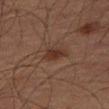{"biopsy_status": "not biopsied; imaged during a skin examination", "image": {"source": "total-body photography crop", "field_of_view_mm": 15}, "lesion_size": {"long_diameter_mm_approx": 3.0}, "lighting": "cross-polarized", "patient": {"sex": "male", "age_approx": 65}, "site": "left thigh"}